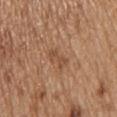{
  "biopsy_status": "not biopsied; imaged during a skin examination",
  "lesion_size": {
    "long_diameter_mm_approx": 3.0
  },
  "patient": {
    "sex": "male",
    "age_approx": 70
  },
  "automated_metrics": {
    "nevus_likeness_0_100": 0
  },
  "image": {
    "source": "total-body photography crop",
    "field_of_view_mm": 15
  },
  "site": "head or neck"
}